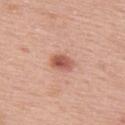Impression: The lesion was tiled from a total-body skin photograph and was not biopsied. Background: The subject is a male aged 53 to 57. Measured at roughly 3 mm in maximum diameter. Located on the upper back. This is a white-light tile. A close-up tile cropped from a whole-body skin photograph, about 15 mm across.